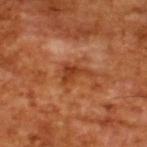Imaged during a routine full-body skin examination; the lesion was not biopsied and no histopathology is available. A roughly 15 mm field-of-view crop from a total-body skin photograph. A male subject, in their mid-60s. Automated image analysis of the tile measured a lesion area of about 6 mm², an outline eccentricity of about 0.8 (0 = round, 1 = elongated), and a shape-asymmetry score of about 0.55 (0 = symmetric). And it measured a mean CIELAB color near L≈41 a*≈28 b*≈37, roughly 9 lightness units darker than nearby skin, and a normalized border contrast of about 7.5. It also reported a lesion-detection confidence of about 100/100. This is a cross-polarized tile. Longest diameter approximately 4 mm.